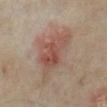Impression:
No biopsy was performed on this lesion — it was imaged during a full skin examination and was not determined to be concerning.
Context:
On the left lower leg. A region of skin cropped from a whole-body photographic capture, roughly 15 mm wide. The tile uses cross-polarized illumination. Approximately 7.5 mm at its widest. A female subject about 35 years old.